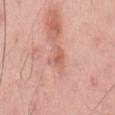The lesion was tiled from a total-body skin photograph and was not biopsied.
A male subject, in their mid-60s.
Cropped from a total-body skin-imaging series; the visible field is about 15 mm.
The lesion is on the back.